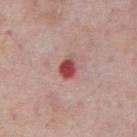notes: total-body-photography surveillance lesion; no biopsy.